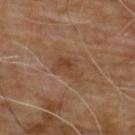Q: Where on the body is the lesion?
A: the upper back
Q: What is the imaging modality?
A: 15 mm crop, total-body photography
Q: Patient demographics?
A: male, roughly 65 years of age